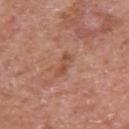Case summary:
* follow-up · total-body-photography surveillance lesion; no biopsy
* lesion size · ~3 mm (longest diameter)
* site · the left upper arm
* patient · male, aged 63 to 67
* image source · ~15 mm tile from a whole-body skin photo
* illumination · white-light illumination